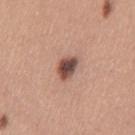Clinical impression: Recorded during total-body skin imaging; not selected for excision or biopsy. Clinical summary: A female patient aged 28–32. About 3.5 mm across. A 15 mm close-up extracted from a 3D total-body photography capture. The lesion is on the leg.